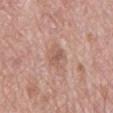Clinical impression:
Imaged during a routine full-body skin examination; the lesion was not biopsied and no histopathology is available.
Clinical summary:
A male subject, aged around 70. From the mid back. Measured at roughly 4 mm in maximum diameter. Imaged with white-light lighting. This image is a 15 mm lesion crop taken from a total-body photograph.